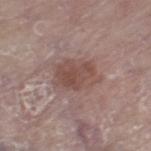Q: Was a biopsy performed?
A: no biopsy performed (imaged during a skin exam)
Q: How was this image acquired?
A: total-body-photography crop, ~15 mm field of view
Q: Lesion location?
A: the left thigh
Q: Patient demographics?
A: female, aged approximately 85 or older